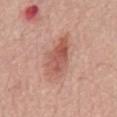Impression:
Recorded during total-body skin imaging; not selected for excision or biopsy.
Acquisition and patient details:
A male subject, aged 68 to 72. Imaged with white-light lighting. An algorithmic analysis of the crop reported a footprint of about 13 mm², an eccentricity of roughly 0.9, and a symmetry-axis asymmetry near 0.25. It also reported a nevus-likeness score of about 95/100 and a lesion-detection confidence of about 100/100. A close-up tile cropped from a whole-body skin photograph, about 15 mm across. Approximately 5.5 mm at its widest. Located on the mid back.